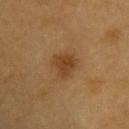Captured during whole-body skin photography for melanoma surveillance; the lesion was not biopsied. Cropped from a total-body skin-imaging series; the visible field is about 15 mm. A male subject about 60 years old. On the back.